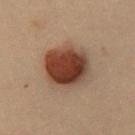imaging modality = 15 mm crop, total-body photography; patient = female, approximately 30 years of age; site = the chest; illumination = cross-polarized; diameter = ~5.5 mm (longest diameter).